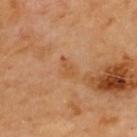The lesion was tiled from a total-body skin photograph and was not biopsied. Imaged with cross-polarized lighting. A male patient aged approximately 70. Located on the upper back. A 15 mm crop from a total-body photograph taken for skin-cancer surveillance. Measured at roughly 3 mm in maximum diameter.